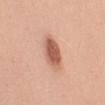Longest diameter approximately 4 mm.
On the arm.
Automated image analysis of the tile measured an eccentricity of roughly 0.7 and a symmetry-axis asymmetry near 0.15. It also reported an average lesion color of about L≈59 a*≈25 b*≈31 (CIELAB), a lesion–skin lightness drop of about 14, and a normalized border contrast of about 9. It also reported a border-irregularity rating of about 1.5/10 and a peripheral color-asymmetry measure near 1.
A female patient in their mid- to late 40s.
A close-up tile cropped from a whole-body skin photograph, about 15 mm across.
Imaged with white-light lighting.